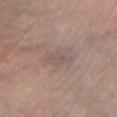Recorded during total-body skin imaging; not selected for excision or biopsy.
The subject is a male roughly 60 years of age.
A region of skin cropped from a whole-body photographic capture, roughly 15 mm wide.
About 4 mm across.
The lesion is located on the leg.
This is a cross-polarized tile.